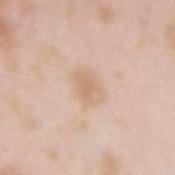Part of a total-body skin-imaging series; this lesion was reviewed on a skin check and was not flagged for biopsy.
About 4 mm across.
A female patient in their mid- to late 20s.
Cropped from a total-body skin-imaging series; the visible field is about 15 mm.
Imaged with white-light lighting.
From the right forearm.